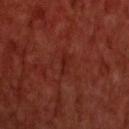Q: Was a biopsy performed?
A: total-body-photography surveillance lesion; no biopsy
Q: What is the anatomic site?
A: the upper back
Q: How was this image acquired?
A: total-body-photography crop, ~15 mm field of view
Q: Who is the patient?
A: male, aged approximately 60